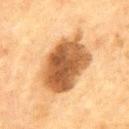No biopsy was performed on this lesion — it was imaged during a full skin examination and was not determined to be concerning. A 15 mm close-up extracted from a 3D total-body photography capture. Located on the chest. About 7.5 mm across. A male patient, roughly 65 years of age.